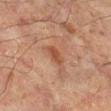Cropped from a whole-body photographic skin survey; the tile spans about 15 mm. Automated image analysis of the tile measured a lesion-detection confidence of about 100/100. About 2.5 mm across. A male subject, in their mid- to late 60s. Located on the left lower leg.